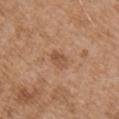This lesion was catalogued during total-body skin photography and was not selected for biopsy. Captured under white-light illumination. The lesion is located on the right upper arm. A 15 mm close-up tile from a total-body photography series done for melanoma screening. The total-body-photography lesion software estimated a lesion area of about 3.5 mm². And it measured an average lesion color of about L≈52 a*≈21 b*≈33 (CIELAB), a lesion–skin lightness drop of about 8, and a normalized lesion–skin contrast near 6. A male subject, aged 73 to 77.